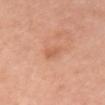This lesion was catalogued during total-body skin photography and was not selected for biopsy. Automated image analysis of the tile measured a mean CIELAB color near L≈60 a*≈25 b*≈35 and a normalized border contrast of about 5. The analysis additionally found a nevus-likeness score of about 0/100 and lesion-presence confidence of about 100/100. A female patient, aged approximately 50. Approximately 2.5 mm at its widest. Cropped from a whole-body photographic skin survey; the tile spans about 15 mm. Located on the chest.